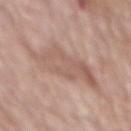{"biopsy_status": "not biopsied; imaged during a skin examination", "automated_metrics": {"area_mm2_approx": 20.0, "eccentricity": 0.75, "shape_asymmetry": 0.4, "cielab_L": 58, "cielab_a": 18, "cielab_b": 25, "vs_skin_darker_L": 8.0, "vs_skin_contrast_norm": 5.0}, "lesion_size": {"long_diameter_mm_approx": 7.0}, "image": {"source": "total-body photography crop", "field_of_view_mm": 15}, "site": "back", "patient": {"sex": "male", "age_approx": 80}}